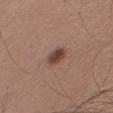Notes:
* workup · catalogued during a skin exam; not biopsied
* size · about 3 mm
* tile lighting · white-light
* body site · the right thigh
* acquisition · ~15 mm tile from a whole-body skin photo
* TBP lesion metrics · an eccentricity of roughly 0.8 and a symmetry-axis asymmetry near 0.15; a lesion color around L≈43 a*≈19 b*≈24 in CIELAB, a lesion–skin lightness drop of about 12, and a normalized border contrast of about 9.5; a nevus-likeness score of about 95/100 and a lesion-detection confidence of about 100/100
* subject · male, aged 48–52